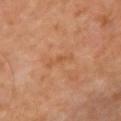Assessment:
The lesion was tiled from a total-body skin photograph and was not biopsied.
Image and clinical context:
Automated image analysis of the tile measured an outline eccentricity of about 0.95 (0 = round, 1 = elongated). The lesion is on the chest. A 15 mm crop from a total-body photograph taken for skin-cancer surveillance. About 3.5 mm across. A female patient, aged approximately 50. The tile uses cross-polarized illumination.